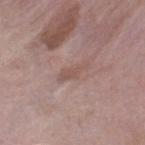Captured during whole-body skin photography for melanoma surveillance; the lesion was not biopsied. An algorithmic analysis of the crop reported a border-irregularity rating of about 6/10, internal color variation of about 0 on a 0–10 scale, and peripheral color asymmetry of about 0. The lesion is located on the right lower leg. A 15 mm crop from a total-body photograph taken for skin-cancer surveillance. The lesion's longest dimension is about 3.5 mm. This is a white-light tile. A male patient, approximately 40 years of age.